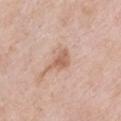A female subject, in their 60s. Cropped from a whole-body photographic skin survey; the tile spans about 15 mm. The tile uses white-light illumination. An algorithmic analysis of the crop reported a lesion area of about 5.5 mm², an outline eccentricity of about 0.75 (0 = round, 1 = elongated), and a shape-asymmetry score of about 0.4 (0 = symmetric). And it measured an average lesion color of about L≈62 a*≈20 b*≈31 (CIELAB), about 10 CIELAB-L* units darker than the surrounding skin, and a normalized border contrast of about 7. It also reported a border-irregularity rating of about 4/10, a color-variation rating of about 2.5/10, and radial color variation of about 0.5. And it measured a detector confidence of about 100 out of 100 that the crop contains a lesion. Located on the front of the torso. About 3.5 mm across.